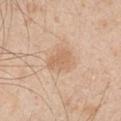notes: no biopsy performed (imaged during a skin exam); site: the left upper arm; patient: male, roughly 50 years of age; acquisition: 15 mm crop, total-body photography.